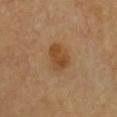Q: What is the anatomic site?
A: the chest
Q: Illumination type?
A: cross-polarized
Q: Who is the patient?
A: male, roughly 55 years of age
Q: How large is the lesion?
A: about 3.5 mm
Q: Automated lesion metrics?
A: a border-irregularity rating of about 2/10, internal color variation of about 4 on a 0–10 scale, and a peripheral color-asymmetry measure near 1.5
Q: What kind of image is this?
A: 15 mm crop, total-body photography